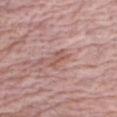Recorded during total-body skin imaging; not selected for excision or biopsy. A roughly 15 mm field-of-view crop from a total-body skin photograph. The tile uses white-light illumination. Located on the right forearm. A male patient roughly 75 years of age.